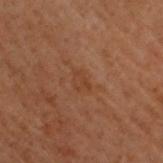Clinical impression:
No biopsy was performed on this lesion — it was imaged during a full skin examination and was not determined to be concerning.
Acquisition and patient details:
The lesion is on the head or neck. A male subject, in their mid- to late 50s. Cropped from a total-body skin-imaging series; the visible field is about 15 mm. Imaged with cross-polarized lighting.